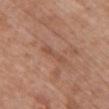Q: Was this lesion biopsied?
A: imaged on a skin check; not biopsied
Q: How was the tile lit?
A: white-light illumination
Q: Lesion size?
A: about 4 mm
Q: What are the patient's age and sex?
A: female, roughly 60 years of age
Q: Where on the body is the lesion?
A: the chest
Q: How was this image acquired?
A: ~15 mm crop, total-body skin-cancer survey